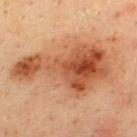Imaged during a routine full-body skin examination; the lesion was not biopsied and no histopathology is available. A male patient approximately 35 years of age. A lesion tile, about 15 mm wide, cut from a 3D total-body photograph. The lesion is located on the upper back.